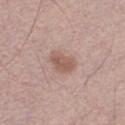Imaged during a routine full-body skin examination; the lesion was not biopsied and no histopathology is available. Automated tile analysis of the lesion measured roughly 9 lightness units darker than nearby skin and a normalized border contrast of about 7. The lesion's longest dimension is about 3.5 mm. Captured under white-light illumination. The subject is a male about 30 years old. A lesion tile, about 15 mm wide, cut from a 3D total-body photograph.